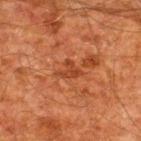| key | value |
|---|---|
| body site | the upper back |
| imaging modality | 15 mm crop, total-body photography |
| lesion size | about 3 mm |
| tile lighting | cross-polarized illumination |
| subject | male, aged 58 to 62 |